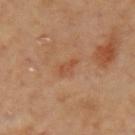anatomic site = the arm; acquisition = 15 mm crop, total-body photography; subject = female, in their mid- to late 60s.